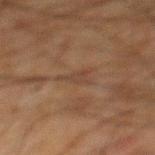  biopsy_status: not biopsied; imaged during a skin examination
  patient:
    sex: male
    age_approx: 65
  image:
    source: total-body photography crop
    field_of_view_mm: 15
  site: mid back
  lesion_size:
    long_diameter_mm_approx: 3.0
  automated_metrics:
    area_mm2_approx: 3.0
    shape_asymmetry: 0.6
    border_irregularity_0_10: 6.5
    color_variation_0_10: 0.5